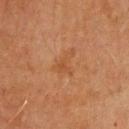This lesion was catalogued during total-body skin photography and was not selected for biopsy. The subject is a male aged around 70. On the upper back. A roughly 15 mm field-of-view crop from a total-body skin photograph.